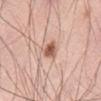Case summary:
– biopsy status: no biopsy performed (imaged during a skin exam)
– subject: male, aged 58–62
– location: the abdomen
– image source: ~15 mm crop, total-body skin-cancer survey